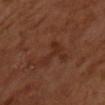{"biopsy_status": "not biopsied; imaged during a skin examination", "lesion_size": {"long_diameter_mm_approx": 3.0}, "lighting": "cross-polarized", "image": {"source": "total-body photography crop", "field_of_view_mm": 15}, "patient": {"sex": "male", "age_approx": 65}}